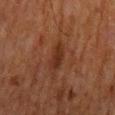Part of a total-body skin-imaging series; this lesion was reviewed on a skin check and was not flagged for biopsy.
Imaged with cross-polarized lighting.
A close-up tile cropped from a whole-body skin photograph, about 15 mm across.
The recorded lesion diameter is about 3.5 mm.
On the back.
A male patient aged around 80.